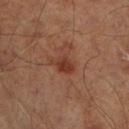biopsy_status: not biopsied; imaged during a skin examination
lighting: cross-polarized
patient:
  sex: male
  age_approx: 65
lesion_size:
  long_diameter_mm_approx: 2.5
site: right lower leg
image:
  source: total-body photography crop
  field_of_view_mm: 15